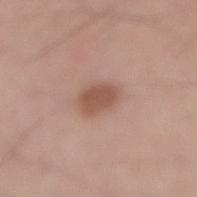{
  "image": {
    "source": "total-body photography crop",
    "field_of_view_mm": 15
  },
  "site": "lower back",
  "patient": {
    "sex": "male",
    "age_approx": 70
  }
}